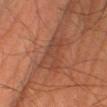imaging modality: 15 mm crop, total-body photography
anatomic site: the left lower leg
patient: male, approximately 65 years of age